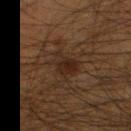workup = imaged on a skin check; not biopsied
location = the right lower leg
lesion size = ≈3.5 mm
subject = male, approximately 35 years of age
image source = ~15 mm crop, total-body skin-cancer survey